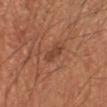No biopsy was performed on this lesion — it was imaged during a full skin examination and was not determined to be concerning.
Automated image analysis of the tile measured a footprint of about 3.5 mm² and an eccentricity of roughly 0.8. The software also gave an average lesion color of about L≈40 a*≈22 b*≈29 (CIELAB), roughly 7 lightness units darker than nearby skin, and a lesion-to-skin contrast of about 6 (normalized; higher = more distinct). It also reported border irregularity of about 3.5 on a 0–10 scale, a within-lesion color-variation index near 1/10, and radial color variation of about 0.5. The analysis additionally found an automated nevus-likeness rating near 25 out of 100 and a detector confidence of about 100 out of 100 that the crop contains a lesion.
The patient is a male aged approximately 55.
Measured at roughly 2.5 mm in maximum diameter.
Captured under cross-polarized illumination.
This image is a 15 mm lesion crop taken from a total-body photograph.
On the left forearm.